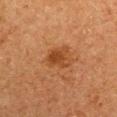{"biopsy_status": "not biopsied; imaged during a skin examination", "patient": {"sex": "male", "age_approx": 75}, "lesion_size": {"long_diameter_mm_approx": 3.0}, "automated_metrics": {"cielab_L": 36, "cielab_a": 22, "cielab_b": 33, "vs_skin_darker_L": 8.0}, "site": "right upper arm", "lighting": "cross-polarized", "image": {"source": "total-body photography crop", "field_of_view_mm": 15}}